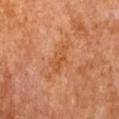– notes: imaged on a skin check; not biopsied
– site: the chest
– automated metrics: an outline eccentricity of about 0.9 (0 = round, 1 = elongated); a border-irregularity rating of about 3.5/10, a color-variation rating of about 3.5/10, and radial color variation of about 1
– tile lighting: cross-polarized
– image: ~15 mm tile from a whole-body skin photo
– subject: male, approximately 85 years of age or older
– lesion diameter: ~4 mm (longest diameter)